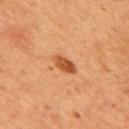Assessment: The lesion was tiled from a total-body skin photograph and was not biopsied. Clinical summary: Automated tile analysis of the lesion measured a footprint of about 4.5 mm², a shape eccentricity near 0.85, and a symmetry-axis asymmetry near 0.2. The recorded lesion diameter is about 3.5 mm. Captured under cross-polarized illumination. Located on the mid back. A 15 mm crop from a total-body photograph taken for skin-cancer surveillance. A male subject, aged 53–57.